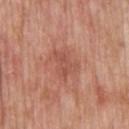Q: Was a biopsy performed?
A: catalogued during a skin exam; not biopsied
Q: What kind of image is this?
A: ~15 mm crop, total-body skin-cancer survey
Q: Lesion location?
A: the upper back
Q: What are the patient's age and sex?
A: male, roughly 60 years of age
Q: What did automated image analysis measure?
A: an area of roughly 6 mm², an outline eccentricity of about 0.75 (0 = round, 1 = elongated), and two-axis asymmetry of about 0.35; a mean CIELAB color near L≈52 a*≈26 b*≈30, roughly 7 lightness units darker than nearby skin, and a normalized lesion–skin contrast near 5.5; lesion-presence confidence of about 100/100
Q: What is the lesion's diameter?
A: ~3.5 mm (longest diameter)
Q: What lighting was used for the tile?
A: white-light